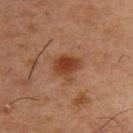The lesion was tiled from a total-body skin photograph and was not biopsied.
On the upper back.
The tile uses cross-polarized illumination.
The recorded lesion diameter is about 3 mm.
A male subject in their mid-50s.
Cropped from a whole-body photographic skin survey; the tile spans about 15 mm.